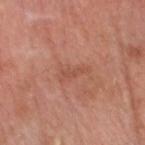No biopsy was performed on this lesion — it was imaged during a full skin examination and was not determined to be concerning. The tile uses white-light illumination. On the head or neck. The patient is a male aged around 80. This image is a 15 mm lesion crop taken from a total-body photograph.